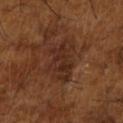Notes:
• workup · imaged on a skin check; not biopsied
• body site · the right upper arm
• subject · male, in their mid- to late 60s
• TBP lesion metrics · a footprint of about 12 mm², a shape eccentricity near 0.85, and two-axis asymmetry of about 0.3; a mean CIELAB color near L≈28 a*≈20 b*≈26 and about 7 CIELAB-L* units darker than the surrounding skin; a nevus-likeness score of about 0/100 and lesion-presence confidence of about 90/100
• image · 15 mm crop, total-body photography
• size · about 6 mm